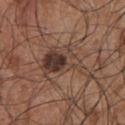notes: imaged on a skin check; not biopsied
patient: male, roughly 55 years of age
acquisition: ~15 mm tile from a whole-body skin photo
body site: the chest